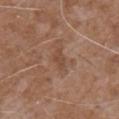Q: What did automated image analysis measure?
A: an area of roughly 2.5 mm², an eccentricity of roughly 0.85, and a symmetry-axis asymmetry near 0.45; a border-irregularity index near 4.5/10 and peripheral color asymmetry of about 0
Q: How was this image acquired?
A: 15 mm crop, total-body photography
Q: Where on the body is the lesion?
A: the back
Q: Illumination type?
A: white-light illumination
Q: Who is the patient?
A: male, aged 58–62